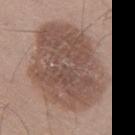notes — no biopsy performed (imaged during a skin exam) | location — the left thigh | automated metrics — a shape eccentricity near 0.7 and a symmetry-axis asymmetry near 0.15; a nevus-likeness score of about 10/100 and a detector confidence of about 100 out of 100 that the crop contains a lesion | imaging modality — 15 mm crop, total-body photography | illumination — white-light | patient — male, aged 58–62.